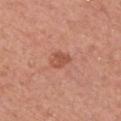Part of a total-body skin-imaging series; this lesion was reviewed on a skin check and was not flagged for biopsy.
Captured under white-light illumination.
On the chest.
The patient is a female in their 60s.
A 15 mm close-up tile from a total-body photography series done for melanoma screening.